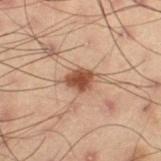• image · 15 mm crop, total-body photography
• anatomic site · the leg
• subject · male, aged 53–57
• lighting · cross-polarized illumination